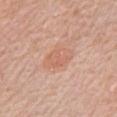The lesion was tiled from a total-body skin photograph and was not biopsied.
A lesion tile, about 15 mm wide, cut from a 3D total-body photograph.
The lesion is on the chest.
Longest diameter approximately 4 mm.
A male patient approximately 80 years of age.